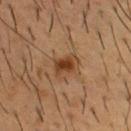Clinical impression:
Captured during whole-body skin photography for melanoma surveillance; the lesion was not biopsied.
Clinical summary:
A 15 mm close-up extracted from a 3D total-body photography capture. From the chest. The patient is a male aged approximately 55.